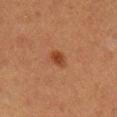Background:
A roughly 15 mm field-of-view crop from a total-body skin photograph. The patient is a female about 40 years old. Automated tile analysis of the lesion measured a border-irregularity index near 1.5/10, a color-variation rating of about 1.5/10, and peripheral color asymmetry of about 0.5. The software also gave lesion-presence confidence of about 100/100. The recorded lesion diameter is about 2.5 mm. The lesion is located on the right lower leg.